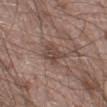biopsy status: catalogued during a skin exam; not biopsied
lighting: white-light
body site: the left lower leg
image: 15 mm crop, total-body photography
subject: male, aged 18 to 22